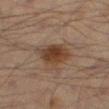Assessment: Part of a total-body skin-imaging series; this lesion was reviewed on a skin check and was not flagged for biopsy. Acquisition and patient details: The subject is a male aged around 70. Measured at roughly 4 mm in maximum diameter. A 15 mm close-up extracted from a 3D total-body photography capture. The lesion is on the right thigh.